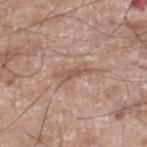The lesion was photographed on a routine skin check and not biopsied; there is no pathology result.
The subject is a male in their 60s.
On the right lower leg.
This is a white-light tile.
This image is a 15 mm lesion crop taken from a total-body photograph.